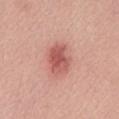Impression: This lesion was catalogued during total-body skin photography and was not selected for biopsy. Image and clinical context: A region of skin cropped from a whole-body photographic capture, roughly 15 mm wide. The subject is a female roughly 65 years of age. Located on the chest. Approximately 4 mm at its widest. Imaged with white-light lighting.